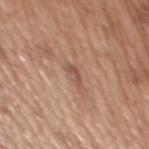workup = imaged on a skin check; not biopsied | TBP lesion metrics = a lesion area of about 2.5 mm², a shape eccentricity near 0.95, and a symmetry-axis asymmetry near 0.4; a border-irregularity rating of about 5/10 and a color-variation rating of about 0/10 | image source = total-body-photography crop, ~15 mm field of view | lesion size = ≈3 mm | location = the mid back | patient = male, aged around 65.